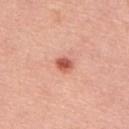Imaged during a routine full-body skin examination; the lesion was not biopsied and no histopathology is available. The lesion is on the back. The subject is a male approximately 60 years of age. A lesion tile, about 15 mm wide, cut from a 3D total-body photograph.